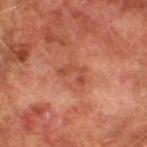Notes:
• biopsy status: imaged on a skin check; not biopsied
• tile lighting: cross-polarized
• diameter: about 3 mm
• patient: male, aged 73–77
• imaging modality: ~15 mm crop, total-body skin-cancer survey
• anatomic site: the left lower leg